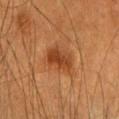{
  "biopsy_status": "not biopsied; imaged during a skin examination",
  "lesion_size": {
    "long_diameter_mm_approx": 4.0
  },
  "patient": {
    "sex": "female",
    "age_approx": 55
  },
  "automated_metrics": {
    "area_mm2_approx": 8.0,
    "eccentricity": 0.65,
    "shape_asymmetry": 0.25,
    "nevus_likeness_0_100": 60,
    "lesion_detection_confidence_0_100": 100
  },
  "image": {
    "source": "total-body photography crop",
    "field_of_view_mm": 15
  },
  "site": "head or neck"
}